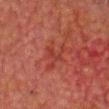Q: Was this lesion biopsied?
A: catalogued during a skin exam; not biopsied
Q: What are the patient's age and sex?
A: male, roughly 80 years of age
Q: Lesion location?
A: the head or neck
Q: How was this image acquired?
A: 15 mm crop, total-body photography